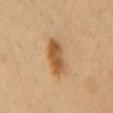Q: Was this lesion biopsied?
A: no biopsy performed (imaged during a skin exam)
Q: What is the anatomic site?
A: the chest
Q: What kind of image is this?
A: ~15 mm tile from a whole-body skin photo
Q: Who is the patient?
A: female, approximately 55 years of age